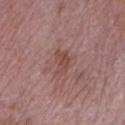workup: no biopsy performed (imaged during a skin exam)
diameter: about 3 mm
imaging modality: total-body-photography crop, ~15 mm field of view
lighting: white-light illumination
patient: female, in their 70s
anatomic site: the left lower leg
automated metrics: an automated nevus-likeness rating near 0 out of 100 and a detector confidence of about 100 out of 100 that the crop contains a lesion